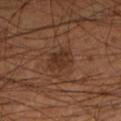<lesion>
<biopsy_status>not biopsied; imaged during a skin examination</biopsy_status>
<image>
  <source>total-body photography crop</source>
  <field_of_view_mm>15</field_of_view_mm>
</image>
<lighting>cross-polarized</lighting>
<lesion_size>
  <long_diameter_mm_approx>3.5</long_diameter_mm_approx>
</lesion_size>
<site>left lower leg</site>
<patient>
  <sex>male</sex>
  <age_approx>55</age_approx>
</patient>
</lesion>